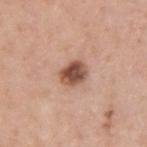  biopsy_status: not biopsied; imaged during a skin examination
  site: back
  image:
    source: total-body photography crop
    field_of_view_mm: 15
  lesion_size:
    long_diameter_mm_approx: 3.0
  patient:
    sex: male
    age_approx: 40
  lighting: white-light